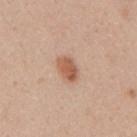A male patient, about 35 years old. From the mid back. A close-up tile cropped from a whole-body skin photograph, about 15 mm across. An algorithmic analysis of the crop reported an eccentricity of roughly 0.8 and a shape-asymmetry score of about 0.2 (0 = symmetric). And it measured a mean CIELAB color near L≈58 a*≈22 b*≈31, about 12 CIELAB-L* units darker than the surrounding skin, and a lesion-to-skin contrast of about 8 (normalized; higher = more distinct). It also reported border irregularity of about 1.5 on a 0–10 scale and a color-variation rating of about 3.5/10. Imaged with white-light lighting.